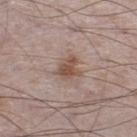Part of a total-body skin-imaging series; this lesion was reviewed on a skin check and was not flagged for biopsy. The total-body-photography lesion software estimated a lesion color around L≈51 a*≈17 b*≈25 in CIELAB and about 10 CIELAB-L* units darker than the surrounding skin. It also reported a border-irregularity index near 3.5/10, a color-variation rating of about 3/10, and peripheral color asymmetry of about 1. It also reported a lesion-detection confidence of about 100/100. Captured under white-light illumination. A male patient, aged around 75. Approximately 3 mm at its widest. A region of skin cropped from a whole-body photographic capture, roughly 15 mm wide. On the left lower leg.